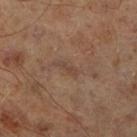Q: What is the imaging modality?
A: 15 mm crop, total-body photography
Q: What are the patient's age and sex?
A: male, aged 63–67
Q: Lesion location?
A: the right lower leg